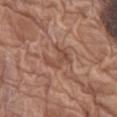Findings:
- follow-up — no biopsy performed (imaged during a skin exam)
- image — ~15 mm crop, total-body skin-cancer survey
- site — the left thigh
- subject — female, roughly 75 years of age
- lesion diameter — ≈4 mm